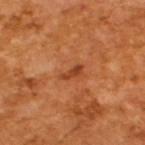{
  "automated_metrics": {
    "eccentricity": 0.9,
    "shape_asymmetry": 0.35,
    "cielab_L": 42,
    "cielab_a": 29,
    "cielab_b": 39,
    "vs_skin_darker_L": 10.0,
    "vs_skin_contrast_norm": 7.5,
    "nevus_likeness_0_100": 0,
    "lesion_detection_confidence_0_100": 100
  },
  "image": {
    "source": "total-body photography crop",
    "field_of_view_mm": 15
  },
  "lighting": "cross-polarized",
  "lesion_size": {
    "long_diameter_mm_approx": 2.5
  },
  "patient": {
    "sex": "male",
    "age_approx": 65
  }
}